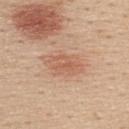Impression: Recorded during total-body skin imaging; not selected for excision or biopsy. Image and clinical context: The lesion is located on the upper back. A close-up tile cropped from a whole-body skin photograph, about 15 mm across. The patient is a female approximately 45 years of age. Imaged with white-light lighting. Approximately 5 mm at its widest.